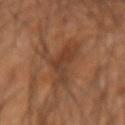{
  "biopsy_status": "not biopsied; imaged during a skin examination",
  "image": {
    "source": "total-body photography crop",
    "field_of_view_mm": 15
  },
  "patient": {
    "sex": "male",
    "age_approx": 45
  },
  "site": "right forearm"
}